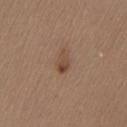The lesion was photographed on a routine skin check and not biopsied; there is no pathology result.
On the back.
Imaged with white-light lighting.
A close-up tile cropped from a whole-body skin photograph, about 15 mm across.
A female patient aged around 40.